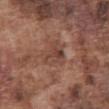follow-up: catalogued during a skin exam; not biopsied | anatomic site: the abdomen | image: ~15 mm tile from a whole-body skin photo | patient: male, aged 73 to 77.